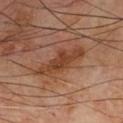notes — catalogued during a skin exam; not biopsied
patient — male, aged around 70
diameter — ~6.5 mm (longest diameter)
lighting — cross-polarized illumination
anatomic site — the chest
image source — 15 mm crop, total-body photography